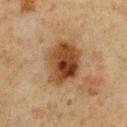workup — no biopsy performed (imaged during a skin exam) | image source — total-body-photography crop, ~15 mm field of view | subject — male, aged around 60 | body site — the front of the torso | tile lighting — cross-polarized illumination | size — about 5.5 mm | image-analysis metrics — a footprint of about 18 mm², a shape eccentricity near 0.65, and two-axis asymmetry of about 0.2; a mean CIELAB color near L≈37 a*≈18 b*≈31, a lesion–skin lightness drop of about 13, and a lesion-to-skin contrast of about 11 (normalized; higher = more distinct).